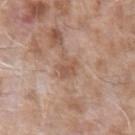follow-up: catalogued during a skin exam; not biopsied.